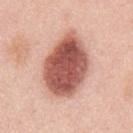notes: imaged on a skin check; not biopsied | image source: ~15 mm tile from a whole-body skin photo | location: the chest | size: ≈7.5 mm | subject: male, in their 50s.